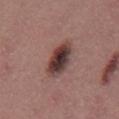Captured during whole-body skin photography for melanoma surveillance; the lesion was not biopsied.
Approximately 4.5 mm at its widest.
Captured under white-light illumination.
From the mid back.
A male patient aged around 40.
A lesion tile, about 15 mm wide, cut from a 3D total-body photograph.